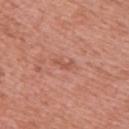This lesion was catalogued during total-body skin photography and was not selected for biopsy. Imaged with white-light lighting. Located on the back. A 15 mm crop from a total-body photograph taken for skin-cancer surveillance. A female patient aged approximately 50.